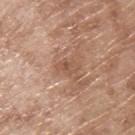Captured during whole-body skin photography for melanoma surveillance; the lesion was not biopsied. Captured under white-light illumination. A male subject aged 63–67. About 2.5 mm across. An algorithmic analysis of the crop reported an area of roughly 3 mm², a shape eccentricity near 0.8, and a symmetry-axis asymmetry near 0.3. It also reported a border-irregularity rating of about 3/10. This image is a 15 mm lesion crop taken from a total-body photograph. From the upper back.